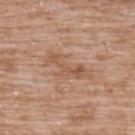Part of a total-body skin-imaging series; this lesion was reviewed on a skin check and was not flagged for biopsy. The subject is a male aged around 50. From the upper back. A close-up tile cropped from a whole-body skin photograph, about 15 mm across. Approximately 5.5 mm at its widest. Captured under white-light illumination.